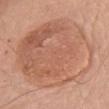This lesion was catalogued during total-body skin photography and was not selected for biopsy.
A 15 mm close-up tile from a total-body photography series done for melanoma screening.
About 11 mm across.
The subject is a male aged 78 to 82.
From the chest.
Captured under white-light illumination.